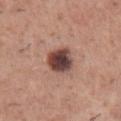A lesion tile, about 15 mm wide, cut from a 3D total-body photograph.
The tile uses white-light illumination.
A male subject about 45 years old.
On the front of the torso.
An algorithmic analysis of the crop reported a mean CIELAB color near L≈43 a*≈21 b*≈22, a lesion–skin lightness drop of about 18, and a normalized lesion–skin contrast near 13. The software also gave a nevus-likeness score of about 75/100 and a detector confidence of about 100 out of 100 that the crop contains a lesion.